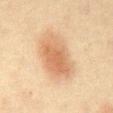notes: imaged on a skin check; not biopsied
imaging modality: 15 mm crop, total-body photography
lesion size: about 7 mm
automated lesion analysis: a border-irregularity index near 1.5/10 and a within-lesion color-variation index near 4/10; an automated nevus-likeness rating near 100 out of 100
subject: male, in their 60s
lighting: cross-polarized
anatomic site: the front of the torso